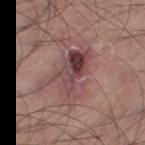follow-up: no biopsy performed (imaged during a skin exam); subject: male, aged 43–47; lesion size: ~6 mm (longest diameter); image source: total-body-photography crop, ~15 mm field of view; body site: the right thigh; automated lesion analysis: an average lesion color of about L≈44 a*≈22 b*≈18 (CIELAB) and about 11 CIELAB-L* units darker than the surrounding skin.